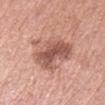About 6.5 mm across. The patient is a female in their mid-60s. The lesion is on the left upper arm. A 15 mm crop from a total-body photograph taken for skin-cancer surveillance. Captured under white-light illumination.